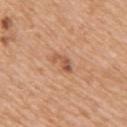Assessment:
Imaged during a routine full-body skin examination; the lesion was not biopsied and no histopathology is available.
Clinical summary:
The patient is a female aged 53 to 57. Located on the right upper arm. A roughly 15 mm field-of-view crop from a total-body skin photograph.